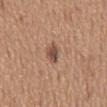{"biopsy_status": "not biopsied; imaged during a skin examination", "patient": {"sex": "male", "age_approx": 65}, "site": "mid back", "image": {"source": "total-body photography crop", "field_of_view_mm": 15}}